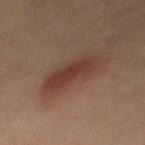Acquisition and patient details: Longest diameter approximately 6.5 mm. A 15 mm close-up tile from a total-body photography series done for melanoma screening. Captured under cross-polarized illumination. From the mid back. A male patient aged 58–62. The total-body-photography lesion software estimated a footprint of about 13 mm², an eccentricity of roughly 0.9, and two-axis asymmetry of about 0.2.